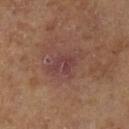The lesion was photographed on a routine skin check and not biopsied; there is no pathology result.
The total-body-photography lesion software estimated a border-irregularity rating of about 4/10, internal color variation of about 3.5 on a 0–10 scale, and peripheral color asymmetry of about 1. And it measured a nevus-likeness score of about 0/100 and lesion-presence confidence of about 100/100.
About 4.5 mm across.
Captured under cross-polarized illumination.
A roughly 15 mm field-of-view crop from a total-body skin photograph.
A male subject, roughly 65 years of age.